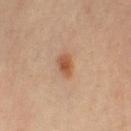Impression:
The lesion was photographed on a routine skin check and not biopsied; there is no pathology result.
Acquisition and patient details:
Automated tile analysis of the lesion measured a footprint of about 4.5 mm², an eccentricity of roughly 0.7, and a shape-asymmetry score of about 0.3 (0 = symmetric). It also reported an automated nevus-likeness rating near 95 out of 100. The subject is a female about 45 years old. This is a cross-polarized tile. A roughly 15 mm field-of-view crop from a total-body skin photograph. Measured at roughly 2.5 mm in maximum diameter. Located on the leg.